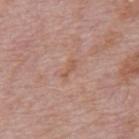Imaged during a routine full-body skin examination; the lesion was not biopsied and no histopathology is available. A male subject in their mid- to late 70s. From the mid back. A roughly 15 mm field-of-view crop from a total-body skin photograph.